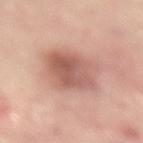biopsy status: catalogued during a skin exam; not biopsied
location: the back
patient: male, roughly 50 years of age
imaging modality: ~15 mm crop, total-body skin-cancer survey